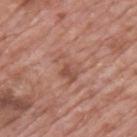The lesion was tiled from a total-body skin photograph and was not biopsied.
A lesion tile, about 15 mm wide, cut from a 3D total-body photograph.
A male patient, roughly 70 years of age.
About 4 mm across.
Located on the upper back.
This is a white-light tile.
Automated image analysis of the tile measured a nevus-likeness score of about 0/100 and a lesion-detection confidence of about 100/100.